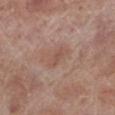Assessment: The lesion was photographed on a routine skin check and not biopsied; there is no pathology result. Background: A male patient, aged 68–72. From the left lower leg. The total-body-photography lesion software estimated border irregularity of about 3 on a 0–10 scale and a color-variation rating of about 2/10. The analysis additionally found an automated nevus-likeness rating near 0 out of 100 and a detector confidence of about 100 out of 100 that the crop contains a lesion. This is a white-light tile. A roughly 15 mm field-of-view crop from a total-body skin photograph.